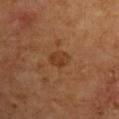This lesion was catalogued during total-body skin photography and was not selected for biopsy.